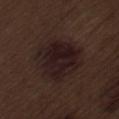Assessment:
The lesion was tiled from a total-body skin photograph and was not biopsied.
Context:
Automated tile analysis of the lesion measured an average lesion color of about L≈17 a*≈14 b*≈13 (CIELAB) and roughly 7 lightness units darker than nearby skin. The analysis additionally found border irregularity of about 3 on a 0–10 scale, a within-lesion color-variation index near 4/10, and peripheral color asymmetry of about 1.5. The software also gave lesion-presence confidence of about 100/100. The lesion is located on the lower back. The tile uses white-light illumination. A 15 mm close-up tile from a total-body photography series done for melanoma screening. Longest diameter approximately 6 mm. A male subject, aged approximately 70.